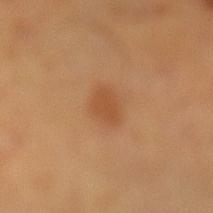Impression:
Recorded during total-body skin imaging; not selected for excision or biopsy.
Image and clinical context:
Imaged with cross-polarized lighting. Cropped from a whole-body photographic skin survey; the tile spans about 15 mm. About 3 mm across. The lesion is on the left lower leg. The subject is a male in their mid-50s.